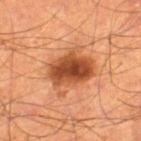follow-up — catalogued during a skin exam; not biopsied.